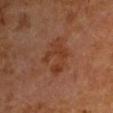lesion_size:
  long_diameter_mm_approx: 4.5
image:
  source: total-body photography crop
  field_of_view_mm: 15
patient:
  sex: male
  age_approx: 65
site: arm